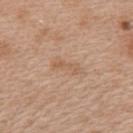Imaged during a routine full-body skin examination; the lesion was not biopsied and no histopathology is available. About 3.5 mm across. The lesion is on the back. The subject is a female aged 38 to 42. Cropped from a whole-body photographic skin survey; the tile spans about 15 mm. This is a white-light tile.